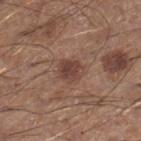| feature | finding |
|---|---|
| notes | catalogued during a skin exam; not biopsied |
| automated lesion analysis | a classifier nevus-likeness of about 60/100 and a detector confidence of about 100 out of 100 that the crop contains a lesion |
| lesion diameter | ≈3 mm |
| subject | male, aged around 60 |
| acquisition | 15 mm crop, total-body photography |
| location | the right lower leg |
| lighting | white-light illumination |